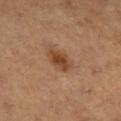workup = imaged on a skin check; not biopsied
location = the right lower leg
subject = female, about 55 years old
acquisition = ~15 mm tile from a whole-body skin photo